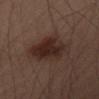Case summary:
• workup · total-body-photography surveillance lesion; no biopsy
• image · ~15 mm tile from a whole-body skin photo
• subject · male, aged 38–42
• lesion size · ≈5.5 mm
• anatomic site · the right thigh
• tile lighting · cross-polarized illumination
• TBP lesion metrics · a color-variation rating of about 4/10 and a peripheral color-asymmetry measure near 1.5; a classifier nevus-likeness of about 95/100